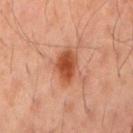The lesion was tiled from a total-body skin photograph and was not biopsied. A roughly 15 mm field-of-view crop from a total-body skin photograph. A male subject about 50 years old. The lesion is on the back. Captured under cross-polarized illumination.